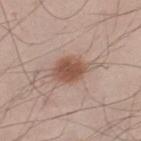Assessment:
Recorded during total-body skin imaging; not selected for excision or biopsy.
Image and clinical context:
The patient is a male approximately 25 years of age. Located on the leg. A region of skin cropped from a whole-body photographic capture, roughly 15 mm wide. This is a white-light tile. The lesion's longest dimension is about 5 mm.